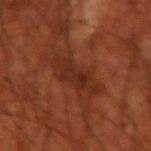<record>
  <biopsy_status>not biopsied; imaged during a skin examination</biopsy_status>
  <patient>
    <sex>male</sex>
    <age_approx>70</age_approx>
  </patient>
  <automated_metrics>
    <area_mm2_approx>10.0</area_mm2_approx>
    <eccentricity>0.95</eccentricity>
    <shape_asymmetry>0.35</shape_asymmetry>
    <cielab_L>28</cielab_L>
    <cielab_a>25</cielab_a>
    <cielab_b>29</cielab_b>
    <vs_skin_darker_L>6.0</vs_skin_darker_L>
    <vs_skin_contrast_norm>6.5</vs_skin_contrast_norm>
    <color_variation_0_10>3.0</color_variation_0_10>
    <peripheral_color_asymmetry>1.0</peripheral_color_asymmetry>
  </automated_metrics>
  <lesion_size>
    <long_diameter_mm_approx>6.0</long_diameter_mm_approx>
  </lesion_size>
  <lighting>cross-polarized</lighting>
  <site>right forearm</site>
  <image>
    <source>total-body photography crop</source>
    <field_of_view_mm>15</field_of_view_mm>
  </image>
</record>